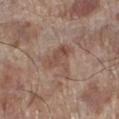<tbp_lesion>
<biopsy_status>not biopsied; imaged during a skin examination</biopsy_status>
<patient>
  <sex>male</sex>
  <age_approx>70</age_approx>
</patient>
<automated_metrics>
  <nevus_likeness_0_100>0</nevus_likeness_0_100>
  <lesion_detection_confidence_0_100>100</lesion_detection_confidence_0_100>
</automated_metrics>
<site>left lower leg</site>
<lighting>white-light</lighting>
<image>
  <source>total-body photography crop</source>
  <field_of_view_mm>15</field_of_view_mm>
</image>
<lesion_size>
  <long_diameter_mm_approx>4.5</long_diameter_mm_approx>
</lesion_size>
</tbp_lesion>